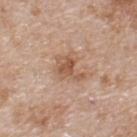Case summary:
* workup · no biopsy performed (imaged during a skin exam)
* automated lesion analysis · a border-irregularity rating of about 5.5/10, internal color variation of about 3 on a 0–10 scale, and peripheral color asymmetry of about 1; a nevus-likeness score of about 0/100
* size · ~4 mm (longest diameter)
* body site · the upper back
* subject · male, approximately 80 years of age
* tile lighting · white-light
* image · total-body-photography crop, ~15 mm field of view